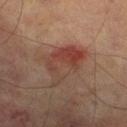{"biopsy_status": "not biopsied; imaged during a skin examination", "lighting": "cross-polarized", "patient": {"sex": "male", "age_approx": 75}, "site": "left lower leg", "image": {"source": "total-body photography crop", "field_of_view_mm": 15}}